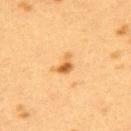{
  "biopsy_status": "not biopsied; imaged during a skin examination",
  "image": {
    "source": "total-body photography crop",
    "field_of_view_mm": 15
  },
  "lesion_size": {
    "long_diameter_mm_approx": 2.5
  },
  "automated_metrics": {
    "area_mm2_approx": 3.5,
    "eccentricity": 0.8,
    "shape_asymmetry": 0.45,
    "border_irregularity_0_10": 4.0,
    "color_variation_0_10": 2.5,
    "peripheral_color_asymmetry": 1.0,
    "nevus_likeness_0_100": 90
  },
  "lighting": "cross-polarized",
  "site": "upper back",
  "patient": {
    "sex": "female",
    "age_approx": 40
  }
}